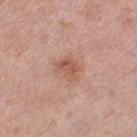Notes:
– follow-up: total-body-photography surveillance lesion; no biopsy
– automated metrics: a footprint of about 6 mm², an outline eccentricity of about 0.6 (0 = round, 1 = elongated), and a shape-asymmetry score of about 0.35 (0 = symmetric); a nevus-likeness score of about 20/100 and a lesion-detection confidence of about 100/100
– tile lighting: white-light illumination
– imaging modality: ~15 mm tile from a whole-body skin photo
– location: the left thigh
– lesion size: ~3 mm (longest diameter)
– subject: female, aged approximately 40